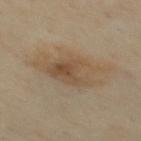Impression: This lesion was catalogued during total-body skin photography and was not selected for biopsy. Image and clinical context: A 15 mm close-up extracted from a 3D total-body photography capture. An algorithmic analysis of the crop reported a footprint of about 19 mm², an eccentricity of roughly 0.8, and two-axis asymmetry of about 0.3. It also reported a nevus-likeness score of about 0/100 and lesion-presence confidence of about 100/100. The subject is a male in their mid-50s. Imaged with cross-polarized lighting. From the back.